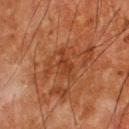Q: Who is the patient?
A: male, aged 63 to 67
Q: What did automated image analysis measure?
A: a mean CIELAB color near L≈32 a*≈23 b*≈30, about 6 CIELAB-L* units darker than the surrounding skin, and a lesion-to-skin contrast of about 6 (normalized; higher = more distinct); border irregularity of about 4.5 on a 0–10 scale, a within-lesion color-variation index near 1.5/10, and peripheral color asymmetry of about 0.5; a classifier nevus-likeness of about 0/100 and lesion-presence confidence of about 100/100
Q: How large is the lesion?
A: ≈3.5 mm
Q: What lighting was used for the tile?
A: cross-polarized
Q: What is the imaging modality?
A: ~15 mm tile from a whole-body skin photo
Q: Where on the body is the lesion?
A: the back